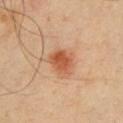This lesion was catalogued during total-body skin photography and was not selected for biopsy. A male subject roughly 40 years of age. Captured under cross-polarized illumination. The lesion's longest dimension is about 3 mm. A close-up tile cropped from a whole-body skin photograph, about 15 mm across. Located on the chest.